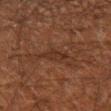follow-up: imaged on a skin check; not biopsied | site: the left forearm | patient: male, aged approximately 50 | acquisition: 15 mm crop, total-body photography | TBP lesion metrics: a footprint of about 3.5 mm² and an outline eccentricity of about 0.75 (0 = round, 1 = elongated); a peripheral color-asymmetry measure near 0.5 | size: about 2.5 mm | tile lighting: cross-polarized.